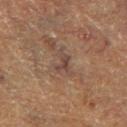Captured during whole-body skin photography for melanoma surveillance; the lesion was not biopsied.
A 15 mm close-up tile from a total-body photography series done for melanoma screening.
Imaged with cross-polarized lighting.
Measured at roughly 3 mm in maximum diameter.
The patient is a male aged 73 to 77.
Located on the left lower leg.